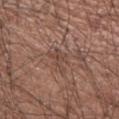follow-up = catalogued during a skin exam; not biopsied
anatomic site = the right upper arm
patient = male, aged approximately 65
acquisition = total-body-photography crop, ~15 mm field of view